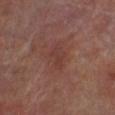Recorded during total-body skin imaging; not selected for excision or biopsy. A male subject aged 68 to 72. An algorithmic analysis of the crop reported an area of roughly 3 mm², a shape eccentricity near 0.9, and a shape-asymmetry score of about 0.35 (0 = symmetric). It also reported a border-irregularity index near 4.5/10, internal color variation of about 0 on a 0–10 scale, and a peripheral color-asymmetry measure near 0. Measured at roughly 3 mm in maximum diameter. Located on the leg. A close-up tile cropped from a whole-body skin photograph, about 15 mm across.